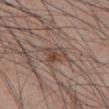<case>
  <biopsy_status>not biopsied; imaged during a skin examination</biopsy_status>
  <patient>
    <sex>male</sex>
    <age_approx>45</age_approx>
  </patient>
  <lighting>white-light</lighting>
  <automated_metrics>
    <border_irregularity_0_10>2.5</border_irregularity_0_10>
    <color_variation_0_10>5.5</color_variation_0_10>
    <nevus_likeness_0_100>10</nevus_likeness_0_100>
    <lesion_detection_confidence_0_100>100</lesion_detection_confidence_0_100>
  </automated_metrics>
  <site>abdomen</site>
  <image>
    <source>total-body photography crop</source>
    <field_of_view_mm>15</field_of_view_mm>
  </image>
  <lesion_size>
    <long_diameter_mm_approx>3.0</long_diameter_mm_approx>
  </lesion_size>
</case>